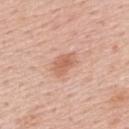  biopsy_status: not biopsied; imaged during a skin examination
  site: upper back
  patient:
    sex: male
    age_approx: 55
  image:
    source: total-body photography crop
    field_of_view_mm: 15
  lighting: white-light
  lesion_size:
    long_diameter_mm_approx: 3.5
  automated_metrics:
    area_mm2_approx: 6.0
    shape_asymmetry: 0.3
    cielab_L: 62
    cielab_a: 24
    cielab_b: 31
    vs_skin_darker_L: 9.0
    vs_skin_contrast_norm: 6.5
    nevus_likeness_0_100: 65